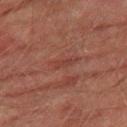No biopsy was performed on this lesion — it was imaged during a full skin examination and was not determined to be concerning.
On the right thigh.
The subject is a male aged 73–77.
Imaged with cross-polarized lighting.
A 15 mm close-up extracted from a 3D total-body photography capture.
The lesion-visualizer software estimated an area of roughly 2.5 mm², an outline eccentricity of about 0.9 (0 = round, 1 = elongated), and a shape-asymmetry score of about 0.3 (0 = symmetric). The software also gave a border-irregularity index near 3.5/10, a color-variation rating of about 0/10, and peripheral color asymmetry of about 0.